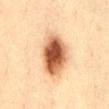<record>
<biopsy_status>not biopsied; imaged during a skin examination</biopsy_status>
<site>abdomen</site>
<lesion_size>
  <long_diameter_mm_approx>6.0</long_diameter_mm_approx>
</lesion_size>
<image>
  <source>total-body photography crop</source>
  <field_of_view_mm>15</field_of_view_mm>
</image>
<patient>
  <sex>female</sex>
  <age_approx>40</age_approx>
</patient>
</record>